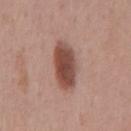illumination — white-light
automated metrics — a lesion area of about 13 mm² and an outline eccentricity of about 0.85 (0 = round, 1 = elongated)
image — 15 mm crop, total-body photography
lesion size — ~5.5 mm (longest diameter)
subject — male, roughly 55 years of age
location — the mid back